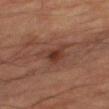follow-up: catalogued during a skin exam; not biopsied | size: ~4.5 mm (longest diameter) | imaging modality: ~15 mm tile from a whole-body skin photo | tile lighting: cross-polarized illumination | body site: the right thigh | TBP lesion metrics: an area of roughly 9 mm², an outline eccentricity of about 0.7 (0 = round, 1 = elongated), and a shape-asymmetry score of about 0.5 (0 = symmetric); roughly 7 lightness units darker than nearby skin; a nevus-likeness score of about 50/100 and a lesion-detection confidence of about 100/100 | subject: male, aged 83 or over.